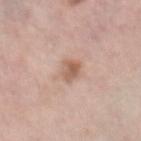Background:
Cropped from a total-body skin-imaging series; the visible field is about 15 mm. A female patient approximately 65 years of age. From the left leg.